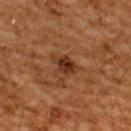| key | value |
|---|---|
| workup | imaged on a skin check; not biopsied |
| image-analysis metrics | an average lesion color of about L≈27 a*≈20 b*≈28 (CIELAB) and a normalized border contrast of about 9; border irregularity of about 3 on a 0–10 scale, a color-variation rating of about 4/10, and peripheral color asymmetry of about 1; an automated nevus-likeness rating near 80 out of 100 and a detector confidence of about 100 out of 100 that the crop contains a lesion |
| acquisition | ~15 mm crop, total-body skin-cancer survey |
| diameter | ≈3 mm |
| body site | the back |
| patient | male, roughly 60 years of age |